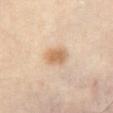| field | value |
|---|---|
| notes | imaged on a skin check; not biopsied |
| anatomic site | the abdomen |
| image source | total-body-photography crop, ~15 mm field of view |
| subject | female, in their 40s |